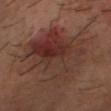Clinical summary:
The lesion's longest dimension is about 8 mm. The tile uses cross-polarized illumination. A male subject aged 48 to 52. From the head or neck. This image is a 15 mm lesion crop taken from a total-body photograph.